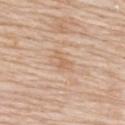notes: imaged on a skin check; not biopsied
image: 15 mm crop, total-body photography
size: ≈3 mm
anatomic site: the upper back
image-analysis metrics: a lesion color around L≈64 a*≈18 b*≈34 in CIELAB and roughly 6 lightness units darker than nearby skin; a nevus-likeness score of about 0/100
patient: female, aged around 70
lighting: white-light